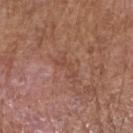The lesion was photographed on a routine skin check and not biopsied; there is no pathology result.
Cropped from a total-body skin-imaging series; the visible field is about 15 mm.
Imaged with white-light lighting.
A male patient in their mid-60s.
Longest diameter approximately 3 mm.
From the right forearm.